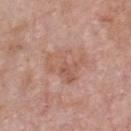Impression: The lesion was tiled from a total-body skin photograph and was not biopsied. Image and clinical context: Cropped from a whole-body photographic skin survey; the tile spans about 15 mm. The lesion is on the chest. A male patient, roughly 55 years of age.